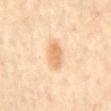Captured during whole-body skin photography for melanoma surveillance; the lesion was not biopsied.
This is a cross-polarized tile.
On the abdomen.
A male subject, approximately 60 years of age.
Cropped from a whole-body photographic skin survey; the tile spans about 15 mm.